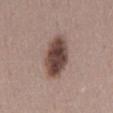Impression: No biopsy was performed on this lesion — it was imaged during a full skin examination and was not determined to be concerning. Acquisition and patient details: A roughly 15 mm field-of-view crop from a total-body skin photograph. The patient is a female approximately 45 years of age. On the back.